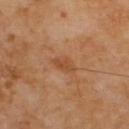Impression: Part of a total-body skin-imaging series; this lesion was reviewed on a skin check and was not flagged for biopsy. Context: A male patient, about 70 years old. Cropped from a whole-body photographic skin survey; the tile spans about 15 mm. From the upper back. Captured under cross-polarized illumination.